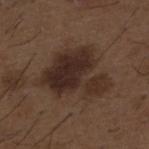biopsy status = imaged on a skin check; not biopsied
image = ~15 mm crop, total-body skin-cancer survey
anatomic site = the upper back
patient = male, about 50 years old
size = ≈7.5 mm
automated metrics = an area of roughly 25 mm² and two-axis asymmetry of about 0.45; a nevus-likeness score of about 60/100 and a lesion-detection confidence of about 100/100